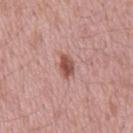The lesion was photographed on a routine skin check and not biopsied; there is no pathology result.
The total-body-photography lesion software estimated a lesion area of about 4 mm², an eccentricity of roughly 0.75, and a symmetry-axis asymmetry near 0.3. And it measured border irregularity of about 2.5 on a 0–10 scale and a within-lesion color-variation index near 3.5/10.
The patient is a male roughly 60 years of age.
Cropped from a total-body skin-imaging series; the visible field is about 15 mm.
Imaged with white-light lighting.
Longest diameter approximately 2.5 mm.
Located on the mid back.